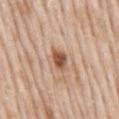| feature | finding |
|---|---|
| workup | catalogued during a skin exam; not biopsied |